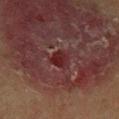follow-up: total-body-photography surveillance lesion; no biopsy
subject: male, approximately 65 years of age
imaging modality: total-body-photography crop, ~15 mm field of view
site: the right lower leg
diameter: ≈2.5 mm
image-analysis metrics: a lesion area of about 4 mm², a shape eccentricity near 0.55, and a symmetry-axis asymmetry near 0.25; a mean CIELAB color near L≈20 a*≈23 b*≈17 and roughly 7 lightness units darker than nearby skin; internal color variation of about 4 on a 0–10 scale; a classifier nevus-likeness of about 0/100 and lesion-presence confidence of about 50/100
lighting: cross-polarized illumination